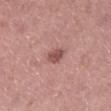<lesion>
  <biopsy_status>not biopsied; imaged during a skin examination</biopsy_status>
  <site>left lower leg</site>
  <image>
    <source>total-body photography crop</source>
    <field_of_view_mm>15</field_of_view_mm>
  </image>
  <lesion_size>
    <long_diameter_mm_approx>2.5</long_diameter_mm_approx>
  </lesion_size>
  <patient>
    <sex>male</sex>
    <age_approx>40</age_approx>
  </patient>
</lesion>